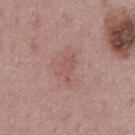Findings:
* notes: catalogued during a skin exam; not biopsied
* acquisition: 15 mm crop, total-body photography
* patient: male, aged 73–77
* automated metrics: a lesion color around L≈53 a*≈23 b*≈23 in CIELAB and a lesion–skin lightness drop of about 6; border irregularity of about 5 on a 0–10 scale, a color-variation rating of about 1/10, and peripheral color asymmetry of about 0.5
* diameter: about 3 mm
* illumination: white-light illumination
* site: the abdomen